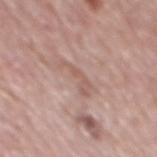Part of a total-body skin-imaging series; this lesion was reviewed on a skin check and was not flagged for biopsy.
The patient is a male aged 68–72.
The lesion's longest dimension is about 4 mm.
A roughly 15 mm field-of-view crop from a total-body skin photograph.
From the mid back.
Captured under white-light illumination.